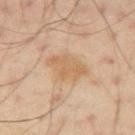Assessment: The lesion was photographed on a routine skin check and not biopsied; there is no pathology result. Context: About 4.5 mm across. The total-body-photography lesion software estimated a mean CIELAB color near L≈62 a*≈17 b*≈35, roughly 7 lightness units darker than nearby skin, and a normalized border contrast of about 6. And it measured an automated nevus-likeness rating near 5 out of 100 and lesion-presence confidence of about 100/100. The subject is a male approximately 50 years of age. A lesion tile, about 15 mm wide, cut from a 3D total-body photograph. From the left arm. Captured under cross-polarized illumination.